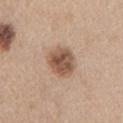Assessment:
Recorded during total-body skin imaging; not selected for excision or biopsy.
Context:
From the left upper arm. The total-body-photography lesion software estimated a mean CIELAB color near L≈54 a*≈18 b*≈29, a lesion–skin lightness drop of about 13, and a normalized border contrast of about 9. The software also gave an automated nevus-likeness rating near 75 out of 100 and lesion-presence confidence of about 100/100. A female patient, aged approximately 45. This is a white-light tile. A close-up tile cropped from a whole-body skin photograph, about 15 mm across.